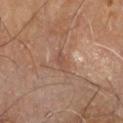No biopsy was performed on this lesion — it was imaged during a full skin examination and was not determined to be concerning. From the right leg. Imaged with cross-polarized lighting. The patient is a male aged around 60. Cropped from a whole-body photographic skin survey; the tile spans about 15 mm.